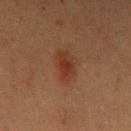notes — imaged on a skin check; not biopsied | location — the left upper arm | imaging modality — total-body-photography crop, ~15 mm field of view | subject — male, aged around 40 | size — about 3.5 mm | TBP lesion metrics — an area of roughly 6 mm², a shape eccentricity near 0.75, and a shape-asymmetry score of about 0.2 (0 = symmetric).